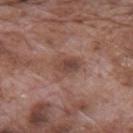  biopsy_status: not biopsied; imaged during a skin examination
  site: mid back
  lighting: white-light
  patient:
    sex: male
    age_approx: 70
  lesion_size:
    long_diameter_mm_approx: 3.5
  image:
    source: total-body photography crop
    field_of_view_mm: 15